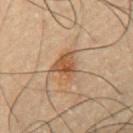Background: Located on the chest. The subject is a male aged approximately 50. About 4 mm across. This is a cross-polarized tile. A region of skin cropped from a whole-body photographic capture, roughly 15 mm wide.